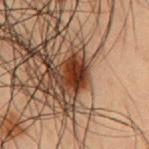Q: Is there a histopathology result?
A: total-body-photography surveillance lesion; no biopsy
Q: How large is the lesion?
A: ~3.5 mm (longest diameter)
Q: What is the imaging modality?
A: 15 mm crop, total-body photography
Q: What is the anatomic site?
A: the chest
Q: What lighting was used for the tile?
A: cross-polarized illumination
Q: Who is the patient?
A: male, aged 53–57